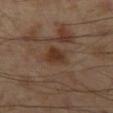No biopsy was performed on this lesion — it was imaged during a full skin examination and was not determined to be concerning. A roughly 15 mm field-of-view crop from a total-body skin photograph. Longest diameter approximately 2.5 mm. On the left thigh. A male patient about 55 years old. Automated image analysis of the tile measured border irregularity of about 2 on a 0–10 scale, a color-variation rating of about 2.5/10, and a peripheral color-asymmetry measure near 0.5.